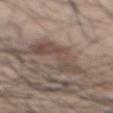Clinical impression: Imaged during a routine full-body skin examination; the lesion was not biopsied and no histopathology is available. Context: Approximately 6.5 mm at its widest. A close-up tile cropped from a whole-body skin photograph, about 15 mm across. On the mid back. The patient is a male approximately 45 years of age.